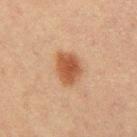Located on the leg. A female subject, about 20 years old. Cropped from a whole-body photographic skin survey; the tile spans about 15 mm.